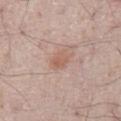biopsy status: total-body-photography surveillance lesion; no biopsy
subject: male, roughly 65 years of age
site: the abdomen
image source: 15 mm crop, total-body photography
illumination: white-light